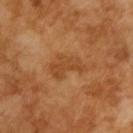The lesion was photographed on a routine skin check and not biopsied; there is no pathology result.
The total-body-photography lesion software estimated a detector confidence of about 100 out of 100 that the crop contains a lesion.
Imaged with cross-polarized lighting.
Approximately 4 mm at its widest.
A male subject, about 65 years old.
This image is a 15 mm lesion crop taken from a total-body photograph.